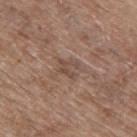notes=no biopsy performed (imaged during a skin exam); acquisition=15 mm crop, total-body photography; patient=male, aged around 70; site=the mid back; image-analysis metrics=a border-irregularity rating of about 6/10, a within-lesion color-variation index near 0/10, and peripheral color asymmetry of about 0; size=~2.5 mm (longest diameter).